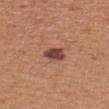notes: no biopsy performed (imaged during a skin exam)
lesion size: about 2.5 mm
patient: male, roughly 55 years of age
image: ~15 mm tile from a whole-body skin photo
tile lighting: white-light illumination
site: the left forearm